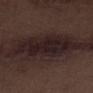Part of a total-body skin-imaging series; this lesion was reviewed on a skin check and was not flagged for biopsy.
Imaged with white-light lighting.
A roughly 15 mm field-of-view crop from a total-body skin photograph.
The lesion is on the right lower leg.
The lesion-visualizer software estimated a mean CIELAB color near L≈19 a*≈13 b*≈13 and roughly 8 lightness units darker than nearby skin. And it measured border irregularity of about 4.5 on a 0–10 scale.
About 8 mm across.
A male subject aged 68 to 72.